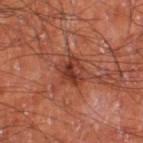Clinical impression:
Imaged during a routine full-body skin examination; the lesion was not biopsied and no histopathology is available.
Acquisition and patient details:
The lesion is located on the leg. The subject is a male roughly 60 years of age. The tile uses cross-polarized illumination. A close-up tile cropped from a whole-body skin photograph, about 15 mm across.